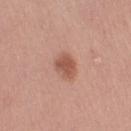Clinical impression: This lesion was catalogued during total-body skin photography and was not selected for biopsy. Clinical summary: Imaged with white-light lighting. This image is a 15 mm lesion crop taken from a total-body photograph. On the left upper arm. A female patient, in their mid- to late 20s. About 3 mm across.